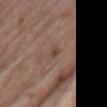Case summary:
- notes — total-body-photography surveillance lesion; no biopsy
- body site — the chest
- acquisition — 15 mm crop, total-body photography
- patient — female, aged approximately 70
- lesion size — ~3 mm (longest diameter)